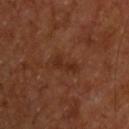The lesion was photographed on a routine skin check and not biopsied; there is no pathology result. The subject is a male approximately 65 years of age. Cropped from a total-body skin-imaging series; the visible field is about 15 mm. The lesion is on the upper back. An algorithmic analysis of the crop reported border irregularity of about 5 on a 0–10 scale and peripheral color asymmetry of about 0.5. Measured at roughly 3.5 mm in maximum diameter. Imaged with cross-polarized lighting.